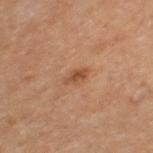follow-up — no biopsy performed (imaged during a skin exam)
lesion size — about 3 mm
body site — the left thigh
subject — male, roughly 65 years of age
acquisition — 15 mm crop, total-body photography